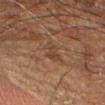Captured during whole-body skin photography for melanoma surveillance; the lesion was not biopsied. The lesion is on the right forearm. About 3.5 mm across. A 15 mm close-up tile from a total-body photography series done for melanoma screening. Imaged with cross-polarized lighting. A male patient, aged around 65.